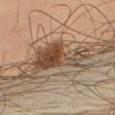body site: the right thigh
imaging modality: ~15 mm tile from a whole-body skin photo
patient: male, approximately 45 years of age